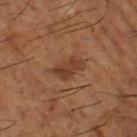notes: total-body-photography surveillance lesion; no biopsy | automated metrics: a mean CIELAB color near L≈39 a*≈22 b*≈32 and roughly 8 lightness units darker than nearby skin; a within-lesion color-variation index near 3/10 and peripheral color asymmetry of about 1 | body site: the leg | lesion diameter: ~4 mm (longest diameter) | lighting: cross-polarized illumination | patient: male, roughly 65 years of age | acquisition: 15 mm crop, total-body photography.